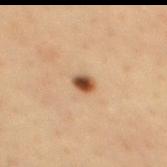The lesion was photographed on a routine skin check and not biopsied; there is no pathology result.